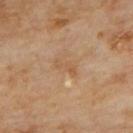| field | value |
|---|---|
| biopsy status | imaged on a skin check; not biopsied |
| TBP lesion metrics | a lesion color around L≈54 a*≈18 b*≈34 in CIELAB, about 6 CIELAB-L* units darker than the surrounding skin, and a lesion-to-skin contrast of about 5 (normalized; higher = more distinct); border irregularity of about 4 on a 0–10 scale; a classifier nevus-likeness of about 0/100 and lesion-presence confidence of about 100/100 |
| body site | the upper back |
| patient | male, about 70 years old |
| imaging modality | ~15 mm tile from a whole-body skin photo |
| lighting | cross-polarized |
| diameter | ≈3 mm |